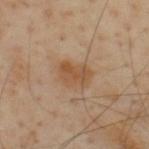{"biopsy_status": "not biopsied; imaged during a skin examination", "lighting": "cross-polarized", "automated_metrics": {"area_mm2_approx": 7.5, "eccentricity": 0.75, "shape_asymmetry": 0.3, "nevus_likeness_0_100": 25, "lesion_detection_confidence_0_100": 100}, "patient": {"sex": "male", "age_approx": 55}, "image": {"source": "total-body photography crop", "field_of_view_mm": 15}, "site": "upper back"}